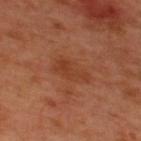Imaged during a routine full-body skin examination; the lesion was not biopsied and no histopathology is available.
The patient is a male aged around 50.
The lesion is on the upper back.
A lesion tile, about 15 mm wide, cut from a 3D total-body photograph.